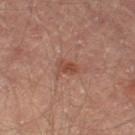Case summary:
– workup · total-body-photography surveillance lesion; no biopsy
– diameter · about 3 mm
– location · the left thigh
– image · ~15 mm tile from a whole-body skin photo
– illumination · cross-polarized
– patient · male, roughly 65 years of age